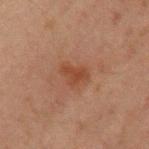Clinical impression: The lesion was photographed on a routine skin check and not biopsied; there is no pathology result. Background: On the arm. A 15 mm close-up tile from a total-body photography series done for melanoma screening. This is a cross-polarized tile. A male patient, roughly 45 years of age. The recorded lesion diameter is about 3 mm.